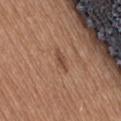The lesion was tiled from a total-body skin photograph and was not biopsied.
The tile uses white-light illumination.
A 15 mm crop from a total-body photograph taken for skin-cancer surveillance.
The lesion is on the back.
A female patient, in their mid-30s.
The lesion's longest dimension is about 2.5 mm.